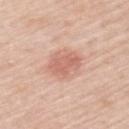  biopsy_status: not biopsied; imaged during a skin examination
  lesion_size:
    long_diameter_mm_approx: 4.0
  site: back
  lighting: white-light
  patient:
    sex: male
    age_approx: 60
  image:
    source: total-body photography crop
    field_of_view_mm: 15
  automated_metrics:
    cielab_L: 63
    cielab_a: 25
    cielab_b: 29
    vs_skin_darker_L: 10.0
    vs_skin_contrast_norm: 6.0
    border_irregularity_0_10: 3.0
    color_variation_0_10: 2.5